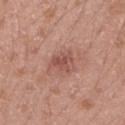workup: imaged on a skin check; not biopsied.